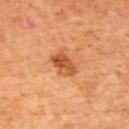Automated image analysis of the tile measured a lesion area of about 6.5 mm² and two-axis asymmetry of about 0.15.
A male subject aged 63–67.
A region of skin cropped from a whole-body photographic capture, roughly 15 mm wide.
Longest diameter approximately 3.5 mm.
Captured under cross-polarized illumination.